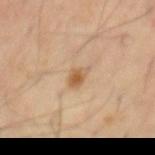Assessment:
The lesion was tiled from a total-body skin photograph and was not biopsied.
Background:
A male subject aged approximately 55. The total-body-photography lesion software estimated a border-irregularity index near 2.5/10 and internal color variation of about 2.5 on a 0–10 scale. The software also gave a classifier nevus-likeness of about 80/100 and lesion-presence confidence of about 100/100. A 15 mm close-up tile from a total-body photography series done for melanoma screening. The lesion is located on the mid back. Captured under cross-polarized illumination.